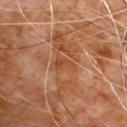Clinical impression:
No biopsy was performed on this lesion — it was imaged during a full skin examination and was not determined to be concerning.
Acquisition and patient details:
Imaged with cross-polarized lighting. A male patient aged approximately 80. The lesion is on the chest. An algorithmic analysis of the crop reported a footprint of about 3 mm², a shape eccentricity near 0.8, and a shape-asymmetry score of about 0.5 (0 = symmetric). The recorded lesion diameter is about 2.5 mm. A region of skin cropped from a whole-body photographic capture, roughly 15 mm wide.